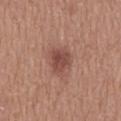Clinical impression: No biopsy was performed on this lesion — it was imaged during a full skin examination and was not determined to be concerning. Clinical summary: From the mid back. An algorithmic analysis of the crop reported an outline eccentricity of about 0.65 (0 = round, 1 = elongated) and two-axis asymmetry of about 0.2. And it measured a border-irregularity index near 2/10 and a peripheral color-asymmetry measure near 1. Approximately 3.5 mm at its widest. The patient is a male aged 63–67. A region of skin cropped from a whole-body photographic capture, roughly 15 mm wide.